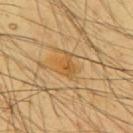Assessment:
Captured during whole-body skin photography for melanoma surveillance; the lesion was not biopsied.
Context:
A male patient, aged around 65. A 15 mm crop from a total-body photograph taken for skin-cancer surveillance. An algorithmic analysis of the crop reported a lesion area of about 6 mm² and a shape eccentricity near 0.6. It also reported a lesion color around L≈58 a*≈19 b*≈45 in CIELAB and roughly 7 lightness units darker than nearby skin. The software also gave border irregularity of about 4.5 on a 0–10 scale, a within-lesion color-variation index near 4/10, and peripheral color asymmetry of about 1.5. The analysis additionally found a classifier nevus-likeness of about 0/100 and a lesion-detection confidence of about 55/100. From the back. The recorded lesion diameter is about 3.5 mm. The tile uses cross-polarized illumination.